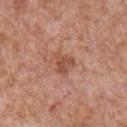Q: Patient demographics?
A: male, aged around 65
Q: How large is the lesion?
A: about 2.5 mm
Q: How was this image acquired?
A: total-body-photography crop, ~15 mm field of view
Q: What lighting was used for the tile?
A: white-light
Q: What is the anatomic site?
A: the chest